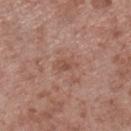Notes:
- workup · no biopsy performed (imaged during a skin exam)
- image source · 15 mm crop, total-body photography
- location · the right lower leg
- diameter · ≈3 mm
- subject · male, aged approximately 55